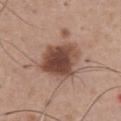notes = imaged on a skin check; not biopsied
size = ~5.5 mm (longest diameter)
acquisition = 15 mm crop, total-body photography
patient = male, roughly 65 years of age
site = the abdomen
illumination = white-light